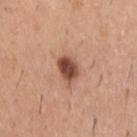Notes:
- workup: no biopsy performed (imaged during a skin exam)
- image: total-body-photography crop, ~15 mm field of view
- subject: male, roughly 60 years of age
- site: the upper back
- tile lighting: white-light
- TBP lesion metrics: border irregularity of about 1.5 on a 0–10 scale
- lesion diameter: about 3 mm